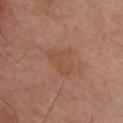Captured during whole-body skin photography for melanoma surveillance; the lesion was not biopsied. A male subject, aged 48–52. Cropped from a whole-body photographic skin survey; the tile spans about 15 mm. On the upper back.